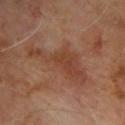The lesion was tiled from a total-body skin photograph and was not biopsied.
A male subject about 65 years old.
The lesion is on the upper back.
Cropped from a total-body skin-imaging series; the visible field is about 15 mm.